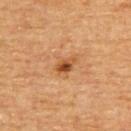Findings:
- biopsy status · imaged on a skin check; not biopsied
- image source · ~15 mm crop, total-body skin-cancer survey
- anatomic site · the upper back
- TBP lesion metrics · an area of roughly 4.5 mm² and an eccentricity of roughly 0.75; a lesion color around L≈47 a*≈22 b*≈37 in CIELAB, roughly 11 lightness units darker than nearby skin, and a normalized lesion–skin contrast near 8; a classifier nevus-likeness of about 65/100 and lesion-presence confidence of about 100/100
- patient · male, aged around 65